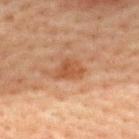No biopsy was performed on this lesion — it was imaged during a full skin examination and was not determined to be concerning.
Measured at roughly 3.5 mm in maximum diameter.
A male patient, approximately 60 years of age.
The tile uses cross-polarized illumination.
Automated image analysis of the tile measured a normalized border contrast of about 7.
From the upper back.
A roughly 15 mm field-of-view crop from a total-body skin photograph.